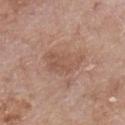Clinical impression: Imaged during a routine full-body skin examination; the lesion was not biopsied and no histopathology is available. Clinical summary: A 15 mm crop from a total-body photograph taken for skin-cancer surveillance. From the chest. Captured under white-light illumination. A male subject aged 63 to 67. Approximately 4.5 mm at its widest.